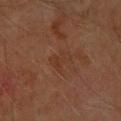Part of a total-body skin-imaging series; this lesion was reviewed on a skin check and was not flagged for biopsy. About 3 mm across. Automated tile analysis of the lesion measured internal color variation of about 2.5 on a 0–10 scale and radial color variation of about 0.5. The analysis additionally found an automated nevus-likeness rating near 0 out of 100 and a lesion-detection confidence of about 100/100. Cropped from a whole-body photographic skin survey; the tile spans about 15 mm. A female patient roughly 70 years of age. Located on the arm.